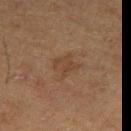workup=imaged on a skin check; not biopsied
tile lighting=cross-polarized
location=the left thigh
diameter=~3 mm (longest diameter)
patient=male, about 75 years old
image source=total-body-photography crop, ~15 mm field of view